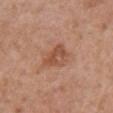{
  "biopsy_status": "not biopsied; imaged during a skin examination",
  "image": {
    "source": "total-body photography crop",
    "field_of_view_mm": 15
  },
  "lighting": "white-light",
  "patient": {
    "sex": "female",
    "age_approx": 70
  },
  "lesion_size": {
    "long_diameter_mm_approx": 3.5
  },
  "site": "chest"
}